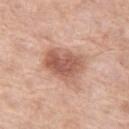Measured at roughly 5 mm in maximum diameter. The tile uses white-light illumination. A close-up tile cropped from a whole-body skin photograph, about 15 mm across. A female subject aged approximately 75. Located on the left thigh.